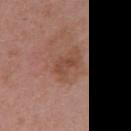Q: Is there a histopathology result?
A: imaged on a skin check; not biopsied
Q: Lesion location?
A: the right upper arm
Q: Lesion size?
A: ~3 mm (longest diameter)
Q: Patient demographics?
A: female, in their mid- to late 40s
Q: How was this image acquired?
A: ~15 mm crop, total-body skin-cancer survey